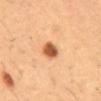• workup · total-body-photography surveillance lesion; no biopsy
• patient · male, aged approximately 50
• diameter · ~2.5 mm (longest diameter)
• tile lighting · cross-polarized
• acquisition · ~15 mm crop, total-body skin-cancer survey
• automated metrics · a lesion area of about 5 mm², an eccentricity of roughly 0.5, and two-axis asymmetry of about 0.15; about 18 CIELAB-L* units darker than the surrounding skin and a normalized lesion–skin contrast near 11; a border-irregularity index near 1.5/10 and a peripheral color-asymmetry measure near 1; a nevus-likeness score of about 100/100 and a lesion-detection confidence of about 100/100
• anatomic site · the abdomen